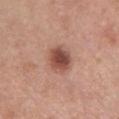Notes:
- notes · imaged on a skin check; not biopsied
- anatomic site · the chest
- image source · ~15 mm tile from a whole-body skin photo
- subject · female, about 55 years old